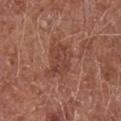Clinical impression:
This lesion was catalogued during total-body skin photography and was not selected for biopsy.
Clinical summary:
Automated image analysis of the tile measured a footprint of about 9 mm², an outline eccentricity of about 0.75 (0 = round, 1 = elongated), and two-axis asymmetry of about 0.25. It also reported border irregularity of about 3.5 on a 0–10 scale, internal color variation of about 3 on a 0–10 scale, and radial color variation of about 1. The analysis additionally found an automated nevus-likeness rating near 5 out of 100 and a detector confidence of about 100 out of 100 that the crop contains a lesion. About 4 mm across. The lesion is located on the abdomen. Cropped from a total-body skin-imaging series; the visible field is about 15 mm. The subject is a male aged approximately 65.